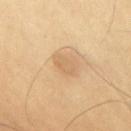This lesion was catalogued during total-body skin photography and was not selected for biopsy. An algorithmic analysis of the crop reported border irregularity of about 2.5 on a 0–10 scale and internal color variation of about 1.5 on a 0–10 scale. The software also gave a nevus-likeness score of about 0/100 and lesion-presence confidence of about 100/100. The lesion is on the chest. Longest diameter approximately 3 mm. A 15 mm close-up tile from a total-body photography series done for melanoma screening. The tile uses cross-polarized illumination. A male subject aged 53–57.